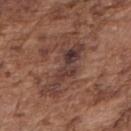follow-up: imaged on a skin check; not biopsied | TBP lesion metrics: an eccentricity of roughly 0.95 and a symmetry-axis asymmetry near 0.55; a border-irregularity index near 9/10 and radial color variation of about 2; a nevus-likeness score of about 25/100 and a lesion-detection confidence of about 85/100 | tile lighting: white-light | anatomic site: the arm | lesion size: about 8 mm | imaging modality: total-body-photography crop, ~15 mm field of view | patient: male, about 75 years old.